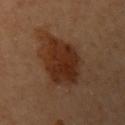Context: Longest diameter approximately 7.5 mm. The patient is a female in their 60s. The lesion is located on the right arm. A region of skin cropped from a whole-body photographic capture, roughly 15 mm wide. Imaged with cross-polarized lighting. An algorithmic analysis of the crop reported a lesion area of about 24 mm² and a shape-asymmetry score of about 0.15 (0 = symmetric). And it measured border irregularity of about 2 on a 0–10 scale and internal color variation of about 4 on a 0–10 scale.